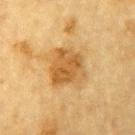<lesion>
<biopsy_status>not biopsied; imaged during a skin examination</biopsy_status>
<lighting>cross-polarized</lighting>
<site>left upper arm</site>
<automated_metrics>
  <eccentricity>0.4</eccentricity>
  <vs_skin_darker_L>10.0</vs_skin_darker_L>
  <vs_skin_contrast_norm>7.5</vs_skin_contrast_norm>
</automated_metrics>
<image>
  <source>total-body photography crop</source>
  <field_of_view_mm>15</field_of_view_mm>
</image>
<patient>
  <sex>male</sex>
  <age_approx>85+</age_approx>
</patient>
</lesion>